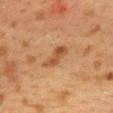follow-up — imaged on a skin check; not biopsied | TBP lesion metrics — a border-irregularity rating of about 3.5/10, a color-variation rating of about 3.5/10, and radial color variation of about 1 | site — the back | image — ~15 mm crop, total-body skin-cancer survey | lesion size — about 4 mm | patient — female, aged approximately 40.